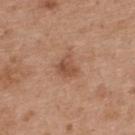– workup · no biopsy performed (imaged during a skin exam)
– anatomic site · the upper back
– image · ~15 mm tile from a whole-body skin photo
– patient · female, aged around 40